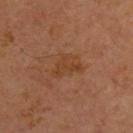The lesion was photographed on a routine skin check and not biopsied; there is no pathology result.
The lesion is located on the left upper arm.
A male patient, aged 63–67.
A region of skin cropped from a whole-body photographic capture, roughly 15 mm wide.
Measured at roughly 4 mm in maximum diameter.
Imaged with cross-polarized lighting.